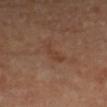{
  "biopsy_status": "not biopsied; imaged during a skin examination",
  "lesion_size": {
    "long_diameter_mm_approx": 3.5
  },
  "automated_metrics": {
    "cielab_L": 40,
    "cielab_a": 20,
    "cielab_b": 29,
    "vs_skin_darker_L": 5.0,
    "vs_skin_contrast_norm": 5.0,
    "color_variation_0_10": 0.0,
    "peripheral_color_asymmetry": 0.0
  },
  "patient": {
    "sex": "female",
    "age_approx": 65
  },
  "lighting": "cross-polarized",
  "image": {
    "source": "total-body photography crop",
    "field_of_view_mm": 15
  },
  "site": "left forearm"
}